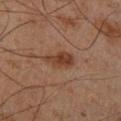Assessment:
The lesion was photographed on a routine skin check and not biopsied; there is no pathology result.
Clinical summary:
The recorded lesion diameter is about 4 mm. Cropped from a whole-body photographic skin survey; the tile spans about 15 mm. Imaged with cross-polarized lighting. The subject is a male roughly 65 years of age. The lesion is on the right lower leg.